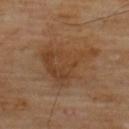A male patient aged around 70. The lesion is on the upper back. The lesion-visualizer software estimated a footprint of about 21 mm², an eccentricity of roughly 0.5, and a shape-asymmetry score of about 0.5 (0 = symmetric). The software also gave a border-irregularity rating of about 6/10, a within-lesion color-variation index near 3.5/10, and a peripheral color-asymmetry measure near 1. The software also gave an automated nevus-likeness rating near 5 out of 100. A lesion tile, about 15 mm wide, cut from a 3D total-body photograph. Imaged with cross-polarized lighting.